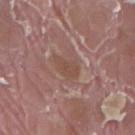<case>
<biopsy_status>not biopsied; imaged during a skin examination</biopsy_status>
<image>
  <source>total-body photography crop</source>
  <field_of_view_mm>15</field_of_view_mm>
</image>
<site>right thigh</site>
<automated_metrics>
  <area_mm2_approx>4.5</area_mm2_approx>
  <eccentricity>0.75</eccentricity>
  <shape_asymmetry>0.3</shape_asymmetry>
  <vs_skin_darker_L>7.0</vs_skin_darker_L>
  <vs_skin_contrast_norm>6.0</vs_skin_contrast_norm>
  <border_irregularity_0_10>3.0</border_irregularity_0_10>
  <color_variation_0_10>1.5</color_variation_0_10>
  <peripheral_color_asymmetry>0.5</peripheral_color_asymmetry>
</automated_metrics>
<patient>
  <sex>male</sex>
  <age_approx>40</age_approx>
</patient>
</case>